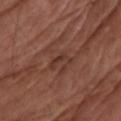Assessment: This lesion was catalogued during total-body skin photography and was not selected for biopsy. Clinical summary: The lesion-visualizer software estimated an area of roughly 3.5 mm² and a symmetry-axis asymmetry near 0.7. The software also gave about 7 CIELAB-L* units darker than the surrounding skin and a lesion-to-skin contrast of about 6 (normalized; higher = more distinct). The analysis additionally found a border-irregularity rating of about 7.5/10, internal color variation of about 0 on a 0–10 scale, and radial color variation of about 0. About 3.5 mm across. Located on the left upper arm. The subject is a male in their mid-70s. Captured under white-light illumination. A 15 mm close-up extracted from a 3D total-body photography capture.